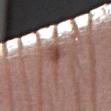<tbp_lesion>
<biopsy_status>not biopsied; imaged during a skin examination</biopsy_status>
<site>leg</site>
<image>
  <source>total-body photography crop</source>
  <field_of_view_mm>15</field_of_view_mm>
</image>
<lesion_size>
  <long_diameter_mm_approx>3.5</long_diameter_mm_approx>
</lesion_size>
<lighting>white-light</lighting>
<automated_metrics>
  <area_mm2_approx>5.5</area_mm2_approx>
  <nevus_likeness_0_100>100</nevus_likeness_0_100>
  <lesion_detection_confidence_0_100>90</lesion_detection_confidence_0_100>
</automated_metrics>
<patient>
  <sex>male</sex>
  <age_approx>65</age_approx>
</patient>
</tbp_lesion>